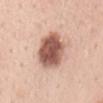{"lesion_size": {"long_diameter_mm_approx": 5.5}, "image": {"source": "total-body photography crop", "field_of_view_mm": 15}, "lighting": "white-light", "site": "back", "patient": {"sex": "female", "age_approx": 45}}